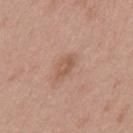This lesion was catalogued during total-body skin photography and was not selected for biopsy. A close-up tile cropped from a whole-body skin photograph, about 15 mm across. Approximately 2.5 mm at its widest. An algorithmic analysis of the crop reported a footprint of about 3 mm² and a symmetry-axis asymmetry near 0.35. The software also gave an average lesion color of about L≈55 a*≈21 b*≈29 (CIELAB). And it measured a border-irregularity rating of about 3/10 and peripheral color asymmetry of about 0.5. And it measured an automated nevus-likeness rating near 25 out of 100 and lesion-presence confidence of about 100/100. A female patient roughly 40 years of age. On the back.